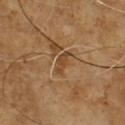Assessment: This lesion was catalogued during total-body skin photography and was not selected for biopsy. Background: Automated tile analysis of the lesion measured border irregularity of about 4 on a 0–10 scale and internal color variation of about 1 on a 0–10 scale. And it measured a nevus-likeness score of about 0/100 and a lesion-detection confidence of about 70/100. A male patient, roughly 60 years of age. Approximately 3.5 mm at its widest. This is a cross-polarized tile. The lesion is on the chest. A close-up tile cropped from a whole-body skin photograph, about 15 mm across.